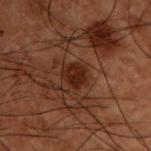Clinical impression:
This lesion was catalogued during total-body skin photography and was not selected for biopsy.
Context:
A 15 mm close-up tile from a total-body photography series done for melanoma screening. The lesion-visualizer software estimated a lesion area of about 5.5 mm², an eccentricity of roughly 0.6, and two-axis asymmetry of about 0.25. The analysis additionally found a lesion color around L≈18 a*≈18 b*≈21 in CIELAB, a lesion–skin lightness drop of about 7, and a normalized lesion–skin contrast near 9.5. The analysis additionally found an automated nevus-likeness rating near 65 out of 100 and a detector confidence of about 100 out of 100 that the crop contains a lesion. From the upper back. The subject is a male aged around 50.